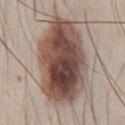Approximately 11 mm at its widest. This is a white-light tile. A male patient, in their mid- to late 40s. The lesion is located on the chest. Cropped from a total-body skin-imaging series; the visible field is about 15 mm.